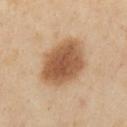This lesion was catalogued during total-body skin photography and was not selected for biopsy. A female subject about 40 years old. A lesion tile, about 15 mm wide, cut from a 3D total-body photograph. From the front of the torso.